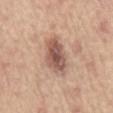Clinical impression: Imaged during a routine full-body skin examination; the lesion was not biopsied and no histopathology is available. Acquisition and patient details: This image is a 15 mm lesion crop taken from a total-body photograph. The lesion's longest dimension is about 5.5 mm. A male subject, about 65 years old. Located on the mid back.